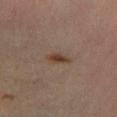<tbp_lesion>
<biopsy_status>not biopsied; imaged during a skin examination</biopsy_status>
<lesion_size>
  <long_diameter_mm_approx>3.0</long_diameter_mm_approx>
</lesion_size>
<patient>
  <sex>male</sex>
  <age_approx>45</age_approx>
</patient>
<image>
  <source>total-body photography crop</source>
  <field_of_view_mm>15</field_of_view_mm>
</image>
<site>right lower leg</site>
<lighting>cross-polarized</lighting>
</tbp_lesion>